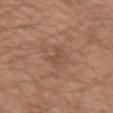Q: Is there a histopathology result?
A: imaged on a skin check; not biopsied
Q: How was this image acquired?
A: ~15 mm tile from a whole-body skin photo
Q: What is the anatomic site?
A: the left upper arm
Q: Lesion size?
A: ~3 mm (longest diameter)
Q: What lighting was used for the tile?
A: white-light
Q: Who is the patient?
A: male, aged around 50